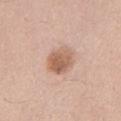biopsy status = catalogued during a skin exam; not biopsied | imaging modality = ~15 mm crop, total-body skin-cancer survey | patient = female, aged around 25 | anatomic site = the chest.